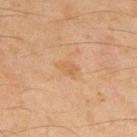<record>
  <biopsy_status>not biopsied; imaged during a skin examination</biopsy_status>
  <site>upper back</site>
  <lighting>cross-polarized</lighting>
  <automated_metrics>
    <nevus_likeness_0_100>0</nevus_likeness_0_100>
  </automated_metrics>
  <image>
    <source>total-body photography crop</source>
    <field_of_view_mm>15</field_of_view_mm>
  </image>
  <patient>
    <sex>male</sex>
    <age_approx>45</age_approx>
  </patient>
</record>